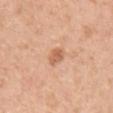Q: Was a biopsy performed?
A: catalogued during a skin exam; not biopsied
Q: Where on the body is the lesion?
A: the arm
Q: How was the tile lit?
A: white-light
Q: How large is the lesion?
A: ~2.5 mm (longest diameter)
Q: What are the patient's age and sex?
A: female, roughly 45 years of age
Q: What is the imaging modality?
A: 15 mm crop, total-body photography
Q: Automated lesion metrics?
A: a classifier nevus-likeness of about 60/100 and a lesion-detection confidence of about 100/100